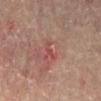Imaged during a routine full-body skin examination; the lesion was not biopsied and no histopathology is available.
A roughly 15 mm field-of-view crop from a total-body skin photograph.
A male subject about 65 years old.
The tile uses cross-polarized illumination.
Measured at roughly 2.5 mm in maximum diameter.
An algorithmic analysis of the crop reported an automated nevus-likeness rating near 0 out of 100 and lesion-presence confidence of about 100/100.
On the front of the torso.